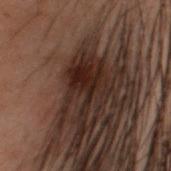Captured during whole-body skin photography for melanoma surveillance; the lesion was not biopsied.
A male patient in their 30s.
A lesion tile, about 15 mm wide, cut from a 3D total-body photograph.
From the head or neck.
The lesion-visualizer software estimated a lesion color around L≈17 a*≈14 b*≈17 in CIELAB, about 9 CIELAB-L* units darker than the surrounding skin, and a normalized border contrast of about 11.5. It also reported internal color variation of about 3 on a 0–10 scale.